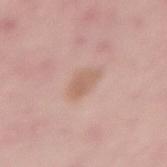Q: Was this lesion biopsied?
A: catalogued during a skin exam; not biopsied
Q: How was the tile lit?
A: white-light
Q: Patient demographics?
A: male, approximately 40 years of age
Q: What kind of image is this?
A: ~15 mm crop, total-body skin-cancer survey
Q: Automated lesion metrics?
A: an area of roughly 4.5 mm² and a shape-asymmetry score of about 0.2 (0 = symmetric); an automated nevus-likeness rating near 5 out of 100 and a lesion-detection confidence of about 100/100
Q: What is the anatomic site?
A: the lower back